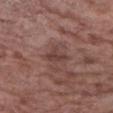No biopsy was performed on this lesion — it was imaged during a full skin examination and was not determined to be concerning. Automated image analysis of the tile measured a lesion area of about 4 mm², a shape eccentricity near 0.75, and two-axis asymmetry of about 0.35. The analysis additionally found a nevus-likeness score of about 0/100 and a lesion-detection confidence of about 95/100. A female patient, aged 68 to 72. Longest diameter approximately 3 mm. Cropped from a whole-body photographic skin survey; the tile spans about 15 mm. The lesion is on the right upper arm.